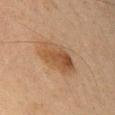follow-up: catalogued during a skin exam; not biopsied
illumination: cross-polarized
imaging modality: ~15 mm tile from a whole-body skin photo
subject: male, aged around 65
anatomic site: the right upper arm
diameter: ≈5.5 mm